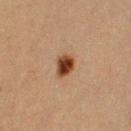Impression: Imaged during a routine full-body skin examination; the lesion was not biopsied and no histopathology is available. Image and clinical context: This image is a 15 mm lesion crop taken from a total-body photograph. This is a cross-polarized tile. The lesion's longest dimension is about 3 mm. A female patient, aged approximately 40. Automated tile analysis of the lesion measured an area of roughly 5 mm², a shape eccentricity near 0.7, and a symmetry-axis asymmetry near 0.2. It also reported an average lesion color of about L≈34 a*≈20 b*≈29 (CIELAB), a lesion–skin lightness drop of about 16, and a normalized lesion–skin contrast near 13.5. The lesion is located on the left upper arm.